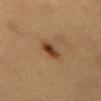notes — no biopsy performed (imaged during a skin exam)
location — the front of the torso
automated lesion analysis — an outline eccentricity of about 0.85 (0 = round, 1 = elongated) and a shape-asymmetry score of about 0.15 (0 = symmetric); an average lesion color of about L≈41 a*≈20 b*≈33 (CIELAB), roughly 12 lightness units darker than nearby skin, and a lesion-to-skin contrast of about 9.5 (normalized; higher = more distinct); a border-irregularity rating of about 1.5/10, a within-lesion color-variation index near 5.5/10, and a peripheral color-asymmetry measure near 2; a classifier nevus-likeness of about 95/100 and lesion-presence confidence of about 100/100
imaging modality — 15 mm crop, total-body photography
patient — male, about 60 years old
tile lighting — cross-polarized illumination
size — ≈3 mm